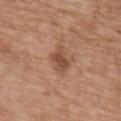No biopsy was performed on this lesion — it was imaged during a full skin examination and was not determined to be concerning. A male subject aged approximately 50. A region of skin cropped from a whole-body photographic capture, roughly 15 mm wide. From the upper back.